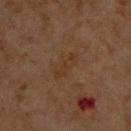Q: Was a biopsy performed?
A: no biopsy performed (imaged during a skin exam)
Q: Lesion location?
A: the chest
Q: Lesion size?
A: ≈4 mm
Q: What is the imaging modality?
A: ~15 mm tile from a whole-body skin photo
Q: Who is the patient?
A: male, about 60 years old
Q: What did automated image analysis measure?
A: a border-irregularity rating of about 3/10, a within-lesion color-variation index near 2/10, and radial color variation of about 0.5; a nevus-likeness score of about 0/100
Q: How was the tile lit?
A: cross-polarized illumination